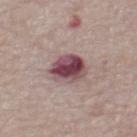This lesion was catalogued during total-body skin photography and was not selected for biopsy.
The subject is a male aged around 75.
An algorithmic analysis of the crop reported a lesion area of about 11 mm² and two-axis asymmetry of about 0.15. The analysis additionally found a nevus-likeness score of about 40/100 and a lesion-detection confidence of about 100/100.
Measured at roughly 4 mm in maximum diameter.
On the back.
A close-up tile cropped from a whole-body skin photograph, about 15 mm across.